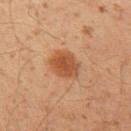Imaged during a routine full-body skin examination; the lesion was not biopsied and no histopathology is available. Longest diameter approximately 4 mm. Cropped from a whole-body photographic skin survey; the tile spans about 15 mm. The lesion-visualizer software estimated a lesion color around L≈46 a*≈23 b*≈34 in CIELAB and a lesion-to-skin contrast of about 8.5 (normalized; higher = more distinct). It also reported border irregularity of about 2 on a 0–10 scale, internal color variation of about 3 on a 0–10 scale, and a peripheral color-asymmetry measure near 1. A male patient, approximately 50 years of age. Located on the arm.